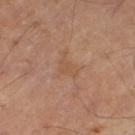Part of a total-body skin-imaging series; this lesion was reviewed on a skin check and was not flagged for biopsy. Imaged with cross-polarized lighting. A 15 mm close-up tile from a total-body photography series done for melanoma screening. On the left thigh. The patient is a male aged around 65.